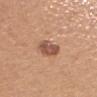biopsy_status: not biopsied; imaged during a skin examination
patient:
  sex: female
  age_approx: 55
site: upper back
image:
  source: total-body photography crop
  field_of_view_mm: 15
automated_metrics:
  area_mm2_approx: 6.5
  shape_asymmetry: 0.2
  border_irregularity_0_10: 2.0
  color_variation_0_10: 2.5
  peripheral_color_asymmetry: 1.0
  nevus_likeness_0_100: 55
  lesion_detection_confidence_0_100: 100
lesion_size:
  long_diameter_mm_approx: 3.5
lighting: white-light